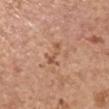Imaged during a routine full-body skin examination; the lesion was not biopsied and no histopathology is available. A female patient in their mid-60s. The lesion is located on the left upper arm. Captured under white-light illumination. Automated image analysis of the tile measured a lesion area of about 4 mm², an eccentricity of roughly 0.9, and two-axis asymmetry of about 0.5. And it measured a lesion color around L≈55 a*≈22 b*≈33 in CIELAB, about 8 CIELAB-L* units darker than the surrounding skin, and a normalized lesion–skin contrast near 5.5. The analysis additionally found border irregularity of about 6.5 on a 0–10 scale, a color-variation rating of about 0/10, and radial color variation of about 0. Measured at roughly 3 mm in maximum diameter. A close-up tile cropped from a whole-body skin photograph, about 15 mm across.